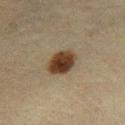<record>
<biopsy_status>not biopsied; imaged during a skin examination</biopsy_status>
</record>